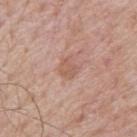Case summary:
* biopsy status — imaged on a skin check; not biopsied
* location — the upper back
* diameter — ≈2.5 mm
* patient — male, aged around 65
* tile lighting — white-light
* image — ~15 mm crop, total-body skin-cancer survey